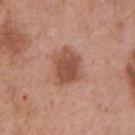| field | value |
|---|---|
| biopsy status | imaged on a skin check; not biopsied |